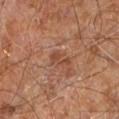Part of a total-body skin-imaging series; this lesion was reviewed on a skin check and was not flagged for biopsy. Located on the leg. The subject is a male aged 58 to 62. A close-up tile cropped from a whole-body skin photograph, about 15 mm across.